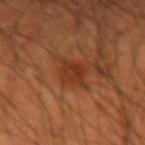The lesion is located on the arm.
About 3.5 mm across.
A 15 mm crop from a total-body photograph taken for skin-cancer surveillance.
The subject is a male about 55 years old.
An algorithmic analysis of the crop reported an area of roughly 6 mm², an outline eccentricity of about 0.75 (0 = round, 1 = elongated), and a symmetry-axis asymmetry near 0.2. It also reported an average lesion color of about L≈29 a*≈22 b*≈29 (CIELAB), roughly 6 lightness units darker than nearby skin, and a normalized lesion–skin contrast near 6.5.